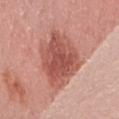follow-up: no biopsy performed (imaged during a skin exam) | lighting: white-light | acquisition: ~15 mm tile from a whole-body skin photo | body site: the left thigh | subject: female, about 40 years old.